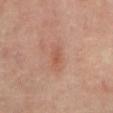{
  "biopsy_status": "not biopsied; imaged during a skin examination",
  "lesion_size": {
    "long_diameter_mm_approx": 2.5
  },
  "lighting": "cross-polarized",
  "patient": {
    "sex": "male",
    "age_approx": 65
  },
  "site": "mid back",
  "image": {
    "source": "total-body photography crop",
    "field_of_view_mm": 15
  }
}